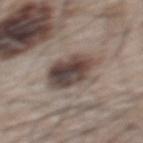No biopsy was performed on this lesion — it was imaged during a full skin examination and was not determined to be concerning. The tile uses white-light illumination. Approximately 6 mm at its widest. The lesion is on the upper back. The patient is a male aged 63 to 67. Cropped from a whole-body photographic skin survey; the tile spans about 15 mm.